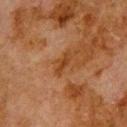This lesion was catalogued during total-body skin photography and was not selected for biopsy. A male subject aged approximately 80. The lesion-visualizer software estimated a border-irregularity index near 4.5/10, internal color variation of about 0 on a 0–10 scale, and radial color variation of about 0. The software also gave a nevus-likeness score of about 0/100 and a detector confidence of about 100 out of 100 that the crop contains a lesion. The tile uses cross-polarized illumination. Located on the upper back. The recorded lesion diameter is about 3 mm. A region of skin cropped from a whole-body photographic capture, roughly 15 mm wide.